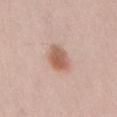Clinical impression:
Recorded during total-body skin imaging; not selected for excision or biopsy.
Clinical summary:
Located on the lower back. A male patient roughly 70 years of age. A region of skin cropped from a whole-body photographic capture, roughly 15 mm wide.